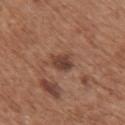Case summary:
- workup: catalogued during a skin exam; not biopsied
- patient: female, aged around 75
- image: ~15 mm tile from a whole-body skin photo
- automated metrics: a lesion color around L≈41 a*≈21 b*≈26 in CIELAB, a lesion–skin lightness drop of about 11, and a lesion-to-skin contrast of about 9 (normalized; higher = more distinct); peripheral color asymmetry of about 1
- body site: the upper back
- illumination: white-light illumination